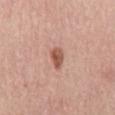The lesion was tiled from a total-body skin photograph and was not biopsied. The patient is a female aged 58 to 62. This is a white-light tile. From the mid back. Cropped from a total-body skin-imaging series; the visible field is about 15 mm.